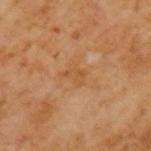notes = catalogued during a skin exam; not biopsied